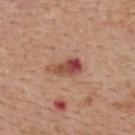biopsy_status: not biopsied; imaged during a skin examination
image:
  source: total-body photography crop
  field_of_view_mm: 15
lighting: white-light
patient:
  sex: male
  age_approx: 65
lesion_size:
  long_diameter_mm_approx: 4.0
site: upper back
automated_metrics:
  area_mm2_approx: 6.0
  eccentricity: 0.9
  shape_asymmetry: 0.35
  cielab_L: 49
  cielab_a: 26
  cielab_b: 29
  vs_skin_darker_L: 14.0
  vs_skin_contrast_norm: 9.5